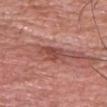Notes:
– biopsy status — imaged on a skin check; not biopsied
– illumination — white-light illumination
– site — the head or neck
– patient — male, aged 53–57
– image source — ~15 mm crop, total-body skin-cancer survey
– size — about 2.5 mm